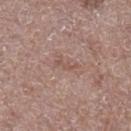Case summary:
- biopsy status · imaged on a skin check; not biopsied
- patient · male, aged around 70
- imaging modality · ~15 mm crop, total-body skin-cancer survey
- site · the leg